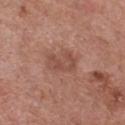Imaged during a routine full-body skin examination; the lesion was not biopsied and no histopathology is available.
A male subject, roughly 80 years of age.
Captured under white-light illumination.
The lesion is located on the chest.
This image is a 15 mm lesion crop taken from a total-body photograph.
The recorded lesion diameter is about 3.5 mm.